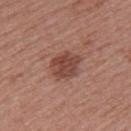Cropped from a total-body skin-imaging series; the visible field is about 15 mm.
Approximately 4 mm at its widest.
A female patient aged 53 to 57.
From the upper back.
Captured under white-light illumination.